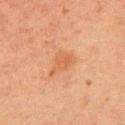Recorded during total-body skin imaging; not selected for excision or biopsy. Automated image analysis of the tile measured a footprint of about 6.5 mm² and a shape-asymmetry score of about 0.4 (0 = symmetric). The software also gave an average lesion color of about L≈49 a*≈21 b*≈33 (CIELAB), roughly 6 lightness units darker than nearby skin, and a normalized border contrast of about 5. And it measured lesion-presence confidence of about 100/100. This is a cross-polarized tile. Longest diameter approximately 4 mm. Cropped from a total-body skin-imaging series; the visible field is about 15 mm. A female subject in their mid- to late 50s. From the arm.